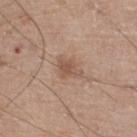{
  "biopsy_status": "not biopsied; imaged during a skin examination",
  "automated_metrics": {
    "area_mm2_approx": 5.0,
    "eccentricity": 0.7,
    "shape_asymmetry": 0.35,
    "cielab_L": 54,
    "cielab_a": 18,
    "cielab_b": 28,
    "peripheral_color_asymmetry": 0.5,
    "nevus_likeness_0_100": 20,
    "lesion_detection_confidence_0_100": 100
  },
  "lighting": "white-light",
  "site": "leg",
  "patient": {
    "sex": "male",
    "age_approx": 65
  },
  "lesion_size": {
    "long_diameter_mm_approx": 3.0
  },
  "image": {
    "source": "total-body photography crop",
    "field_of_view_mm": 15
  }
}